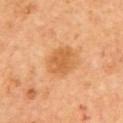A male subject, aged around 65.
Imaged with cross-polarized lighting.
The lesion is on the upper back.
The lesion-visualizer software estimated a lesion area of about 9.5 mm² and an outline eccentricity of about 0.6 (0 = round, 1 = elongated). The software also gave an average lesion color of about L≈61 a*≈25 b*≈44 (CIELAB), about 9 CIELAB-L* units darker than the surrounding skin, and a normalized border contrast of about 6.5. The analysis additionally found a border-irregularity index near 1.5/10, internal color variation of about 2.5 on a 0–10 scale, and radial color variation of about 1. The software also gave lesion-presence confidence of about 100/100.
A lesion tile, about 15 mm wide, cut from a 3D total-body photograph.
Approximately 4 mm at its widest.